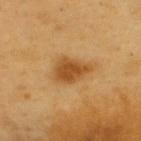Captured during whole-body skin photography for melanoma surveillance; the lesion was not biopsied. Automated image analysis of the tile measured a lesion–skin lightness drop of about 12 and a normalized border contrast of about 8.5. And it measured a detector confidence of about 100 out of 100 that the crop contains a lesion. Cropped from a whole-body photographic skin survey; the tile spans about 15 mm. A male patient aged 58 to 62. On the upper back. The lesion's longest dimension is about 4 mm.